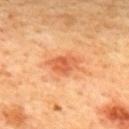Clinical impression: Captured during whole-body skin photography for melanoma surveillance; the lesion was not biopsied. Image and clinical context: Cropped from a whole-body photographic skin survey; the tile spans about 15 mm. The lesion is located on the back. The subject is a male aged around 45.